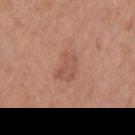Captured during whole-body skin photography for melanoma surveillance; the lesion was not biopsied. A male patient in their 70s. A 15 mm close-up tile from a total-body photography series done for melanoma screening. The lesion is located on the back.